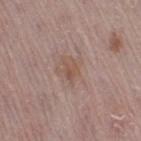<case>
<patient>
  <sex>female</sex>
  <age_approx>50</age_approx>
</patient>
<automated_metrics>
  <area_mm2_approx>3.0</area_mm2_approx>
  <shape_asymmetry>0.4</shape_asymmetry>
  <cielab_L>52</cielab_L>
  <cielab_a>18</cielab_a>
  <cielab_b>26</cielab_b>
  <vs_skin_darker_L>7.0</vs_skin_darker_L>
  <border_irregularity_0_10>4.0</border_irregularity_0_10>
  <color_variation_0_10>1.5</color_variation_0_10>
  <peripheral_color_asymmetry>0.5</peripheral_color_asymmetry>
</automated_metrics>
<site>right thigh</site>
<image>
  <source>total-body photography crop</source>
  <field_of_view_mm>15</field_of_view_mm>
</image>
</case>